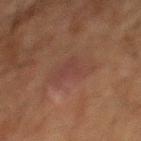workup = no biopsy performed (imaged during a skin exam); site = the mid back; size = ~4.5 mm (longest diameter); image source = 15 mm crop, total-body photography; subject = male, aged 58 to 62.